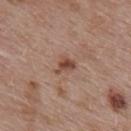Captured during whole-body skin photography for melanoma surveillance; the lesion was not biopsied. Approximately 2.5 mm at its widest. Automated image analysis of the tile measured an area of roughly 3.5 mm² and two-axis asymmetry of about 0.5. The analysis additionally found an average lesion color of about L≈47 a*≈21 b*≈27 (CIELAB), a lesion–skin lightness drop of about 11, and a normalized border contrast of about 8. The analysis additionally found a classifier nevus-likeness of about 30/100 and a detector confidence of about 100 out of 100 that the crop contains a lesion. The lesion is located on the upper back. A female patient, aged 38 to 42. A close-up tile cropped from a whole-body skin photograph, about 15 mm across. This is a white-light tile.